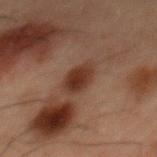Assessment:
Part of a total-body skin-imaging series; this lesion was reviewed on a skin check and was not flagged for biopsy.
Acquisition and patient details:
Cropped from a whole-body photographic skin survey; the tile spans about 15 mm. A male subject, aged around 60. On the mid back. The recorded lesion diameter is about 4 mm. Imaged with cross-polarized lighting.